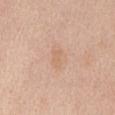Part of a total-body skin-imaging series; this lesion was reviewed on a skin check and was not flagged for biopsy. A lesion tile, about 15 mm wide, cut from a 3D total-body photograph. The patient is a male about 60 years old. Located on the chest. Approximately 2.5 mm at its widest. Automated image analysis of the tile measured a footprint of about 3.5 mm² and an outline eccentricity of about 0.85 (0 = round, 1 = elongated).